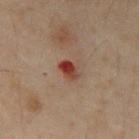notes — imaged on a skin check; not biopsied | imaging modality — total-body-photography crop, ~15 mm field of view | diameter — about 2.5 mm | subject — male, aged around 50 | TBP lesion metrics — a lesion area of about 4 mm², an eccentricity of roughly 0.8, and a symmetry-axis asymmetry near 0.2; a border-irregularity rating of about 2/10, internal color variation of about 8.5 on a 0–10 scale, and radial color variation of about 3.5; a nevus-likeness score of about 0/100 and lesion-presence confidence of about 100/100 | body site — the chest | lighting — cross-polarized.